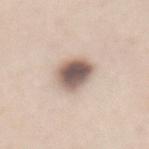Recorded during total-body skin imaging; not selected for excision or biopsy.
This is a white-light tile.
The lesion-visualizer software estimated a footprint of about 11 mm². The analysis additionally found about 19 CIELAB-L* units darker than the surrounding skin and a normalized border contrast of about 11.5. And it measured an automated nevus-likeness rating near 90 out of 100 and lesion-presence confidence of about 100/100.
A female patient, aged around 50.
The lesion is located on the mid back.
The recorded lesion diameter is about 4.5 mm.
A lesion tile, about 15 mm wide, cut from a 3D total-body photograph.